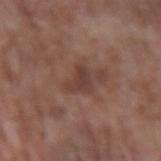| feature | finding |
|---|---|
| biopsy status | imaged on a skin check; not biopsied |
| image | ~15 mm tile from a whole-body skin photo |
| lesion size | ~3 mm (longest diameter) |
| illumination | white-light illumination |
| patient | male, aged 63–67 |
| automated lesion analysis | an area of roughly 5 mm², a shape eccentricity near 0.7, and a shape-asymmetry score of about 0.5 (0 = symmetric); a lesion-to-skin contrast of about 6.5 (normalized; higher = more distinct) |
| location | the left upper arm |